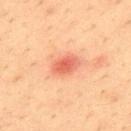The lesion was tiled from a total-body skin photograph and was not biopsied.
On the upper back.
A 15 mm close-up extracted from a 3D total-body photography capture.
Automated tile analysis of the lesion measured a lesion color around L≈60 a*≈31 b*≈34 in CIELAB, a lesion–skin lightness drop of about 11, and a lesion-to-skin contrast of about 7 (normalized; higher = more distinct). It also reported a nevus-likeness score of about 0/100 and lesion-presence confidence of about 100/100.
Longest diameter approximately 3.5 mm.
The tile uses cross-polarized illumination.
The subject is a male in their mid-30s.